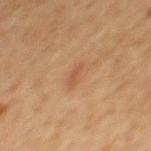Clinical impression: Part of a total-body skin-imaging series; this lesion was reviewed on a skin check and was not flagged for biopsy. Clinical summary: The tile uses cross-polarized illumination. Longest diameter approximately 2.5 mm. The lesion is on the mid back. The total-body-photography lesion software estimated an average lesion color of about L≈46 a*≈20 b*≈30 (CIELAB), a lesion–skin lightness drop of about 6, and a normalized border contrast of about 5. It also reported a border-irregularity index near 2.5/10, a within-lesion color-variation index near 1/10, and peripheral color asymmetry of about 0.5. A male subject in their mid- to late 60s. Cropped from a whole-body photographic skin survey; the tile spans about 15 mm.